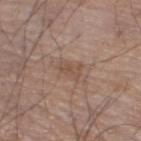Part of a total-body skin-imaging series; this lesion was reviewed on a skin check and was not flagged for biopsy. A male patient, approximately 70 years of age. Cropped from a total-body skin-imaging series; the visible field is about 15 mm. On the right thigh.